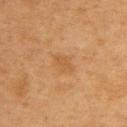Q: Where on the body is the lesion?
A: the upper back
Q: How was the tile lit?
A: cross-polarized
Q: What is the lesion's diameter?
A: about 3 mm
Q: What is the imaging modality?
A: 15 mm crop, total-body photography
Q: Patient demographics?
A: male, aged around 55